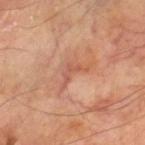workup: no biopsy performed (imaged during a skin exam) | lesion diameter: about 3.5 mm | body site: the left thigh | illumination: cross-polarized illumination | patient: male, aged 68 to 72 | automated metrics: two-axis asymmetry of about 0.75; a border-irregularity rating of about 8.5/10 and a within-lesion color-variation index near 0/10; a lesion-detection confidence of about 60/100 | acquisition: ~15 mm crop, total-body skin-cancer survey.